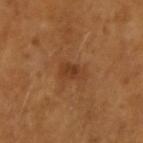Captured under cross-polarized illumination. A region of skin cropped from a whole-body photographic capture, roughly 15 mm wide. Approximately 3 mm at its widest. The subject is a female aged around 55. Located on the right forearm. The lesion-visualizer software estimated an outline eccentricity of about 0.8 (0 = round, 1 = elongated) and a shape-asymmetry score of about 0.3 (0 = symmetric). It also reported a border-irregularity index near 3/10, internal color variation of about 2 on a 0–10 scale, and radial color variation of about 0.5.